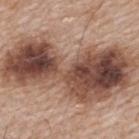Q: Was this lesion biopsied?
A: total-body-photography surveillance lesion; no biopsy
Q: Patient demographics?
A: male, aged around 65
Q: How was the tile lit?
A: white-light
Q: What is the imaging modality?
A: ~15 mm crop, total-body skin-cancer survey
Q: Lesion location?
A: the upper back
Q: Automated lesion metrics?
A: a lesion color around L≈48 a*≈19 b*≈26 in CIELAB; a nevus-likeness score of about 95/100 and a detector confidence of about 100 out of 100 that the crop contains a lesion